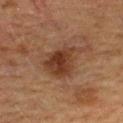The lesion was tiled from a total-body skin photograph and was not biopsied. The patient is a female aged 53 to 57. Cropped from a whole-body photographic skin survey; the tile spans about 15 mm. This is a cross-polarized tile. The lesion is located on the upper back.